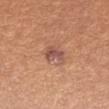Case summary:
* notes · imaged on a skin check; not biopsied
* automated lesion analysis · border irregularity of about 4 on a 0–10 scale, internal color variation of about 3.5 on a 0–10 scale, and a peripheral color-asymmetry measure near 1.5; a detector confidence of about 100 out of 100 that the crop contains a lesion
* anatomic site · the right forearm
* diameter · about 3 mm
* imaging modality · 15 mm crop, total-body photography
* illumination · white-light illumination
* subject · female, about 25 years old